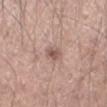Clinical impression: Imaged during a routine full-body skin examination; the lesion was not biopsied and no histopathology is available. Acquisition and patient details: The lesion-visualizer software estimated a lesion color around L≈55 a*≈19 b*≈24 in CIELAB and a normalized border contrast of about 7. And it measured a border-irregularity rating of about 1.5/10, a color-variation rating of about 3.5/10, and a peripheral color-asymmetry measure near 1. A male patient, in their mid-40s. The recorded lesion diameter is about 2.5 mm. On the left forearm. A lesion tile, about 15 mm wide, cut from a 3D total-body photograph.